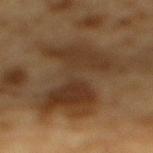Findings:
– biopsy status · imaged on a skin check; not biopsied
– illumination · cross-polarized illumination
– image · ~15 mm tile from a whole-body skin photo
– site · the mid back
– patient · male, approximately 85 years of age or older
– size · about 10 mm